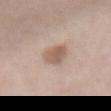{
  "biopsy_status": "not biopsied; imaged during a skin examination",
  "site": "abdomen",
  "image": {
    "source": "total-body photography crop",
    "field_of_view_mm": 15
  },
  "patient": {
    "sex": "female",
    "age_approx": 80
  }
}